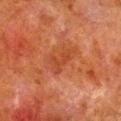This lesion was catalogued during total-body skin photography and was not selected for biopsy. This image is a 15 mm lesion crop taken from a total-body photograph. On the leg. A male subject aged 78 to 82. This is a cross-polarized tile. Longest diameter approximately 4 mm.